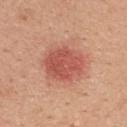<lesion>
<biopsy_status>not biopsied; imaged during a skin examination</biopsy_status>
<patient>
  <sex>female</sex>
  <age_approx>45</age_approx>
</patient>
<site>upper back</site>
<automated_metrics>
  <area_mm2_approx>16.0</area_mm2_approx>
  <eccentricity>0.6</eccentricity>
  <shape_asymmetry>0.15</shape_asymmetry>
  <cielab_L>55</cielab_L>
  <cielab_a>30</cielab_a>
  <cielab_b>30</cielab_b>
  <vs_skin_darker_L>11.0</vs_skin_darker_L>
</automated_metrics>
<lesion_size>
  <long_diameter_mm_approx>5.0</long_diameter_mm_approx>
</lesion_size>
<image>
  <source>total-body photography crop</source>
  <field_of_view_mm>15</field_of_view_mm>
</image>
<lighting>white-light</lighting>
</lesion>